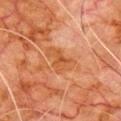biopsy_status: not biopsied; imaged during a skin examination
site: chest
lesion_size:
  long_diameter_mm_approx: 4.5
lighting: cross-polarized
patient:
  sex: male
  age_approx: 80
image:
  source: total-body photography crop
  field_of_view_mm: 15